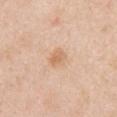Q: Was a biopsy performed?
A: total-body-photography surveillance lesion; no biopsy
Q: Where on the body is the lesion?
A: the right upper arm
Q: What lighting was used for the tile?
A: white-light
Q: What did automated image analysis measure?
A: a lesion-to-skin contrast of about 6 (normalized; higher = more distinct); border irregularity of about 2 on a 0–10 scale, a within-lesion color-variation index near 2/10, and a peripheral color-asymmetry measure near 0.5; a nevus-likeness score of about 25/100
Q: What are the patient's age and sex?
A: female, aged approximately 50
Q: Lesion size?
A: ~2.5 mm (longest diameter)
Q: What kind of image is this?
A: ~15 mm tile from a whole-body skin photo